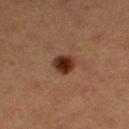This lesion was catalogued during total-body skin photography and was not selected for biopsy.
The lesion is on the left thigh.
The lesion-visualizer software estimated a mean CIELAB color near L≈25 a*≈20 b*≈24, roughly 13 lightness units darker than nearby skin, and a lesion-to-skin contrast of about 13 (normalized; higher = more distinct).
About 2.5 mm across.
This image is a 15 mm lesion crop taken from a total-body photograph.
Imaged with cross-polarized lighting.
A female subject in their 40s.